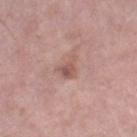The lesion's longest dimension is about 2.5 mm. The patient is a female approximately 60 years of age. The lesion is on the right thigh. A 15 mm close-up tile from a total-body photography series done for melanoma screening. The lesion-visualizer software estimated a footprint of about 4 mm² and two-axis asymmetry of about 0.5. And it measured a mean CIELAB color near L≈55 a*≈21 b*≈24, a lesion–skin lightness drop of about 9, and a normalized lesion–skin contrast near 6.5. The software also gave border irregularity of about 5 on a 0–10 scale, internal color variation of about 3 on a 0–10 scale, and peripheral color asymmetry of about 1. It also reported an automated nevus-likeness rating near 0 out of 100 and a lesion-detection confidence of about 100/100.